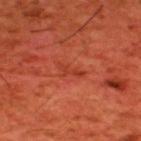The lesion was tiled from a total-body skin photograph and was not biopsied.
From the upper back.
A 15 mm close-up tile from a total-body photography series done for melanoma screening.
A male patient aged around 60.
The recorded lesion diameter is about 3 mm.
The lesion-visualizer software estimated a mean CIELAB color near L≈40 a*≈35 b*≈36 and roughly 6 lightness units darker than nearby skin. The software also gave a border-irregularity index near 5.5/10. The software also gave a nevus-likeness score of about 0/100 and lesion-presence confidence of about 100/100.
The tile uses cross-polarized illumination.